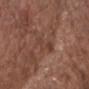Case summary:
– patient · male, aged 73 to 77
– image-analysis metrics · a lesion area of about 4.5 mm² and an eccentricity of roughly 0.9; a mean CIELAB color near L≈40 a*≈20 b*≈26; border irregularity of about 4.5 on a 0–10 scale, a within-lesion color-variation index near 1.5/10, and radial color variation of about 0; an automated nevus-likeness rating near 0 out of 100 and lesion-presence confidence of about 90/100
– size · ~3.5 mm (longest diameter)
– tile lighting · white-light illumination
– acquisition · 15 mm crop, total-body photography
– anatomic site · the chest